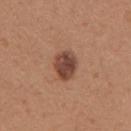The lesion was tiled from a total-body skin photograph and was not biopsied. The patient is a female approximately 45 years of age. From the right upper arm. Longest diameter approximately 3.5 mm. A lesion tile, about 15 mm wide, cut from a 3D total-body photograph.